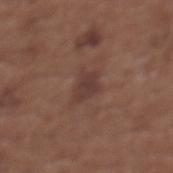<record>
  <biopsy_status>not biopsied; imaged during a skin examination</biopsy_status>
  <lesion_size>
    <long_diameter_mm_approx>3.0</long_diameter_mm_approx>
  </lesion_size>
  <automated_metrics>
    <area_mm2_approx>5.5</area_mm2_approx>
    <eccentricity>0.55</eccentricity>
    <shape_asymmetry>0.3</shape_asymmetry>
    <color_variation_0_10>1.5</color_variation_0_10>
    <nevus_likeness_0_100>0</nevus_likeness_0_100>
    <lesion_detection_confidence_0_100>100</lesion_detection_confidence_0_100>
  </automated_metrics>
  <image>
    <source>total-body photography crop</source>
    <field_of_view_mm>15</field_of_view_mm>
  </image>
  <patient>
    <sex>female</sex>
    <age_approx>65</age_approx>
  </patient>
  <lighting>white-light</lighting>
  <site>upper back</site>
</record>